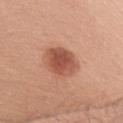follow-up = catalogued during a skin exam; not biopsied
subject = female, aged 38–42
anatomic site = the right upper arm
acquisition = total-body-photography crop, ~15 mm field of view